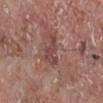Recorded during total-body skin imaging; not selected for excision or biopsy. The tile uses white-light illumination. Automated image analysis of the tile measured a mean CIELAB color near L≈45 a*≈22 b*≈23, roughly 8 lightness units darker than nearby skin, and a lesion-to-skin contrast of about 6.5 (normalized; higher = more distinct). The software also gave a border-irregularity rating of about 6/10, a within-lesion color-variation index near 1.5/10, and a peripheral color-asymmetry measure near 0.5. A 15 mm crop from a total-body photograph taken for skin-cancer surveillance. The subject is a male in their mid- to late 70s. From the right lower leg.